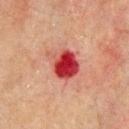workup=no biopsy performed (imaged during a skin exam) | lesion diameter=≈4 mm | automated lesion analysis=a nevus-likeness score of about 0/100 and a lesion-detection confidence of about 100/100 | subject=male, aged 68 to 72 | location=the chest | image=15 mm crop, total-body photography | illumination=cross-polarized illumination.